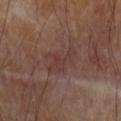notes: no biopsy performed (imaged during a skin exam) | image-analysis metrics: a footprint of about 9 mm², a shape eccentricity near 0.8, and a shape-asymmetry score of about 0.45 (0 = symmetric); a lesion color around L≈38 a*≈19 b*≈22 in CIELAB and a lesion-to-skin contrast of about 4.5 (normalized; higher = more distinct); an automated nevus-likeness rating near 0 out of 100 and lesion-presence confidence of about 100/100 | image source: total-body-photography crop, ~15 mm field of view | lighting: cross-polarized illumination | lesion size: ~4.5 mm (longest diameter) | anatomic site: the left forearm.